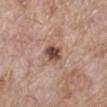Notes:
• follow-up — total-body-photography surveillance lesion; no biopsy
• lighting — white-light
• subject — female, in their 70s
• size — ≈3 mm
• anatomic site — the left lower leg
• image source — 15 mm crop, total-body photography